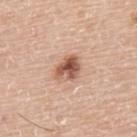Q: Was this lesion biopsied?
A: no biopsy performed (imaged during a skin exam)
Q: Patient demographics?
A: male, aged approximately 75
Q: Lesion size?
A: ≈3.5 mm
Q: What is the imaging modality?
A: 15 mm crop, total-body photography
Q: How was the tile lit?
A: white-light
Q: Where on the body is the lesion?
A: the back